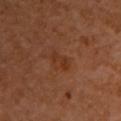  biopsy_status: not biopsied; imaged during a skin examination
  lighting: cross-polarized
  patient:
    sex: female
    age_approx: 55
  site: front of the torso
  automated_metrics:
    eccentricity: 0.9
    shape_asymmetry: 0.45
    vs_skin_darker_L: 6.0
    border_irregularity_0_10: 5.5
    color_variation_0_10: 0.0
    peripheral_color_asymmetry: 0.0
    nevus_likeness_0_100: 5
    lesion_detection_confidence_0_100: 100
  image:
    source: total-body photography crop
    field_of_view_mm: 15